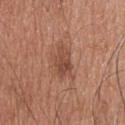workup=no biopsy performed (imaged during a skin exam); subject=male, aged approximately 45; tile lighting=white-light; imaging modality=15 mm crop, total-body photography; location=the upper back.